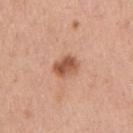Captured during whole-body skin photography for melanoma surveillance; the lesion was not biopsied.
A male patient approximately 55 years of age.
Cropped from a total-body skin-imaging series; the visible field is about 15 mm.
The tile uses white-light illumination.
The lesion-visualizer software estimated a border-irregularity index near 1.5/10, a color-variation rating of about 4.5/10, and radial color variation of about 2. And it measured a nevus-likeness score of about 75/100 and lesion-presence confidence of about 100/100.
Measured at roughly 3 mm in maximum diameter.
Located on the arm.